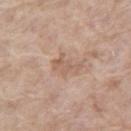follow-up: no biopsy performed (imaged during a skin exam) | site: the right thigh | subject: female, aged approximately 75 | size: ~3.5 mm (longest diameter) | lighting: white-light | image source: ~15 mm crop, total-body skin-cancer survey.